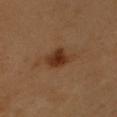<case>
  <biopsy_status>not biopsied; imaged during a skin examination</biopsy_status>
  <patient>
    <sex>male</sex>
    <age_approx>60</age_approx>
  </patient>
  <image>
    <source>total-body photography crop</source>
    <field_of_view_mm>15</field_of_view_mm>
  </image>
  <lighting>cross-polarized</lighting>
  <lesion_size>
    <long_diameter_mm_approx>4.0</long_diameter_mm_approx>
  </lesion_size>
  <site>right upper arm</site>
</case>